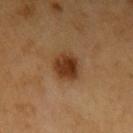Clinical impression: The lesion was tiled from a total-body skin photograph and was not biopsied.